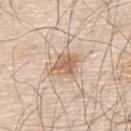• workup: no biopsy performed (imaged during a skin exam)
• imaging modality: ~15 mm crop, total-body skin-cancer survey
• patient: male, aged approximately 80
• automated lesion analysis: a footprint of about 7.5 mm², an outline eccentricity of about 0.6 (0 = round, 1 = elongated), and two-axis asymmetry of about 0.2; internal color variation of about 3.5 on a 0–10 scale
• lighting: white-light illumination
• location: the upper back